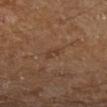Q: Was this lesion biopsied?
A: total-body-photography surveillance lesion; no biopsy
Q: How large is the lesion?
A: ~2.5 mm (longest diameter)
Q: Patient demographics?
A: male, approximately 60 years of age
Q: Lesion location?
A: the left lower leg
Q: What is the imaging modality?
A: ~15 mm tile from a whole-body skin photo
Q: Automated lesion metrics?
A: a classifier nevus-likeness of about 0/100 and lesion-presence confidence of about 100/100
Q: What lighting was used for the tile?
A: cross-polarized illumination